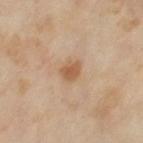Assessment: Imaged during a routine full-body skin examination; the lesion was not biopsied and no histopathology is available. Background: On the left thigh. The subject is a female approximately 50 years of age. Imaged with cross-polarized lighting. The recorded lesion diameter is about 2.5 mm. Automated image analysis of the tile measured a lesion area of about 4 mm², an eccentricity of roughly 0.55, and a shape-asymmetry score of about 0.25 (0 = symmetric). It also reported a mean CIELAB color near L≈56 a*≈19 b*≈34. And it measured border irregularity of about 2 on a 0–10 scale, internal color variation of about 1.5 on a 0–10 scale, and peripheral color asymmetry of about 0.5. It also reported a classifier nevus-likeness of about 75/100 and a detector confidence of about 100 out of 100 that the crop contains a lesion. A close-up tile cropped from a whole-body skin photograph, about 15 mm across.